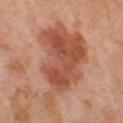Q: Was this lesion biopsied?
A: no biopsy performed (imaged during a skin exam)
Q: What are the patient's age and sex?
A: female, about 50 years old
Q: How large is the lesion?
A: ≈10.5 mm
Q: Lesion location?
A: the left thigh
Q: How was this image acquired?
A: total-body-photography crop, ~15 mm field of view